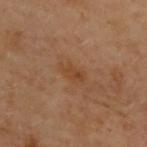workup: no biopsy performed (imaged during a skin exam) | lesion diameter: ≈3 mm | imaging modality: 15 mm crop, total-body photography | anatomic site: the upper back | subject: female, roughly 60 years of age | tile lighting: cross-polarized | automated lesion analysis: a lesion area of about 4 mm², an eccentricity of roughly 0.85, and a symmetry-axis asymmetry near 0.25; a mean CIELAB color near L≈37 a*≈18 b*≈31, a lesion–skin lightness drop of about 5, and a normalized border contrast of about 6; a nevus-likeness score of about 0/100 and a lesion-detection confidence of about 100/100.